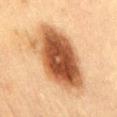Q: Was a biopsy performed?
A: no biopsy performed (imaged during a skin exam)
Q: Where on the body is the lesion?
A: the abdomen
Q: What did automated image analysis measure?
A: an outline eccentricity of about 0.8 (0 = round, 1 = elongated) and a shape-asymmetry score of about 0.2 (0 = symmetric); a border-irregularity index near 2.5/10 and a within-lesion color-variation index near 9/10
Q: What is the imaging modality?
A: ~15 mm tile from a whole-body skin photo
Q: How large is the lesion?
A: ≈9.5 mm
Q: How was the tile lit?
A: cross-polarized
Q: What are the patient's age and sex?
A: male, in their 60s